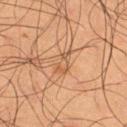Assessment: Part of a total-body skin-imaging series; this lesion was reviewed on a skin check and was not flagged for biopsy. Clinical summary: The tile uses cross-polarized illumination. A 15 mm close-up tile from a total-body photography series done for melanoma screening. The patient is a male about 50 years old. An algorithmic analysis of the crop reported a classifier nevus-likeness of about 0/100 and a lesion-detection confidence of about 60/100. The lesion's longest dimension is about 3.5 mm. The lesion is located on the right thigh.